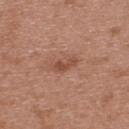{"lesion_size": {"long_diameter_mm_approx": 2.5}, "patient": {"sex": "female", "age_approx": 40}, "site": "upper back", "image": {"source": "total-body photography crop", "field_of_view_mm": 15}, "automated_metrics": {"border_irregularity_0_10": 4.5, "peripheral_color_asymmetry": 0.0}}